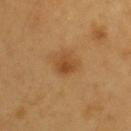Findings:
- illumination — cross-polarized
- lesion size — about 2.5 mm
- subject — male, aged 58–62
- automated metrics — a lesion color around L≈45 a*≈22 b*≈39 in CIELAB and a lesion-to-skin contrast of about 7 (normalized; higher = more distinct); a border-irregularity rating of about 2.5/10, a color-variation rating of about 2.5/10, and radial color variation of about 0.5; a detector confidence of about 100 out of 100 that the crop contains a lesion
- anatomic site — the back
- acquisition — 15 mm crop, total-body photography